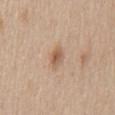| feature | finding |
|---|---|
| lighting | white-light |
| body site | the chest |
| lesion size | ≈3 mm |
| image source | 15 mm crop, total-body photography |
| subject | male, in their 60s |